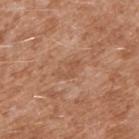A lesion tile, about 15 mm wide, cut from a 3D total-body photograph.
The lesion is located on the right upper arm.
Longest diameter approximately 3 mm.
The patient is a male approximately 45 years of age.